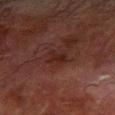<record>
  <biopsy_status>not biopsied; imaged during a skin examination</biopsy_status>
  <image>
    <source>total-body photography crop</source>
    <field_of_view_mm>15</field_of_view_mm>
  </image>
  <lesion_size>
    <long_diameter_mm_approx>2.5</long_diameter_mm_approx>
  </lesion_size>
  <site>left forearm</site>
  <patient>
    <sex>male</sex>
    <age_approx>80</age_approx>
  </patient>
  <lighting>cross-polarized</lighting>
  <automated_metrics>
    <area_mm2_approx>3.0</area_mm2_approx>
    <eccentricity>0.75</eccentricity>
    <shape_asymmetry>0.5</shape_asymmetry>
    <border_irregularity_0_10>5.5</border_irregularity_0_10>
    <color_variation_0_10>0.5</color_variation_0_10>
    <peripheral_color_asymmetry>0.0</peripheral_color_asymmetry>
  </automated_metrics>
</record>